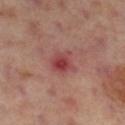Findings:
– notes: total-body-photography surveillance lesion; no biopsy
– automated metrics: a footprint of about 6 mm², a shape eccentricity near 0.55, and a symmetry-axis asymmetry near 0.3
– patient: female, aged 53 to 57
– site: the leg
– illumination: cross-polarized illumination
– imaging modality: 15 mm crop, total-body photography